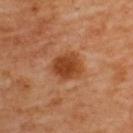On the upper back.
A female subject in their mid-60s.
Imaged with cross-polarized lighting.
A close-up tile cropped from a whole-body skin photograph, about 15 mm across.
Measured at roughly 4 mm in maximum diameter.